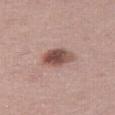Findings:
– workup — total-body-photography surveillance lesion; no biopsy